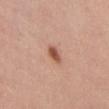| field | value |
|---|---|
| follow-up | imaged on a skin check; not biopsied |
| body site | the chest |
| lesion diameter | about 2.5 mm |
| subject | male, aged around 60 |
| acquisition | total-body-photography crop, ~15 mm field of view |